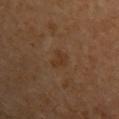  biopsy_status: not biopsied; imaged during a skin examination
  automated_metrics:
    area_mm2_approx: 3.5
    eccentricity: 0.8
    shape_asymmetry: 0.35
    color_variation_0_10: 1.0
    peripheral_color_asymmetry: 0.5
    lesion_detection_confidence_0_100: 100
  patient:
    sex: female
    age_approx: 50
  image:
    source: total-body photography crop
    field_of_view_mm: 15
  lesion_size:
    long_diameter_mm_approx: 2.5
  site: left upper arm